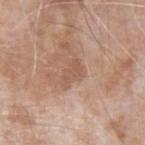| field | value |
|---|---|
| workup | catalogued during a skin exam; not biopsied |
| automated lesion analysis | a lesion area of about 4.5 mm², an eccentricity of roughly 0.8, and a shape-asymmetry score of about 0.4 (0 = symmetric); a lesion color around L≈55 a*≈20 b*≈30 in CIELAB, roughly 7 lightness units darker than nearby skin, and a normalized border contrast of about 5; a nevus-likeness score of about 0/100 |
| imaging modality | 15 mm crop, total-body photography |
| diameter | ≈3 mm |
| tile lighting | white-light illumination |
| location | the arm |
| patient | male, about 75 years old |